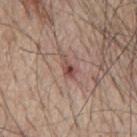Clinical impression:
This lesion was catalogued during total-body skin photography and was not selected for biopsy.
Background:
The tile uses white-light illumination. A lesion tile, about 15 mm wide, cut from a 3D total-body photograph. A male subject aged 63–67. The lesion-visualizer software estimated a lesion color around L≈48 a*≈22 b*≈22 in CIELAB, roughly 11 lightness units darker than nearby skin, and a lesion-to-skin contrast of about 8 (normalized; higher = more distinct). The software also gave a border-irregularity rating of about 5.5/10. The software also gave a classifier nevus-likeness of about 0/100 and a detector confidence of about 100 out of 100 that the crop contains a lesion. The lesion is located on the back. Longest diameter approximately 3 mm.